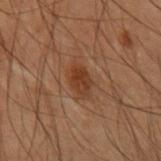- image — 15 mm crop, total-body photography
- location — the right forearm
- diameter — about 3.5 mm
- patient — male, in their mid- to late 50s
- lighting — cross-polarized